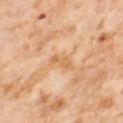biopsy status=catalogued during a skin exam; not biopsied
tile lighting=cross-polarized illumination
image=15 mm crop, total-body photography
diameter=~3 mm (longest diameter)
subject=female, approximately 55 years of age
automated metrics=border irregularity of about 5 on a 0–10 scale; a classifier nevus-likeness of about 0/100 and a detector confidence of about 100 out of 100 that the crop contains a lesion
site=the leg